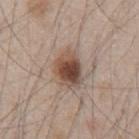This lesion was catalogued during total-body skin photography and was not selected for biopsy. Located on the abdomen. The total-body-photography lesion software estimated a lesion area of about 10 mm² and an outline eccentricity of about 0.7 (0 = round, 1 = elongated). The software also gave an average lesion color of about L≈47 a*≈17 b*≈25 (CIELAB) and about 15 CIELAB-L* units darker than the surrounding skin. The analysis additionally found border irregularity of about 2 on a 0–10 scale and a peripheral color-asymmetry measure near 2. The software also gave lesion-presence confidence of about 100/100. The patient is a male in their mid-40s. A 15 mm crop from a total-body photograph taken for skin-cancer surveillance. Approximately 4 mm at its widest. The tile uses white-light illumination.